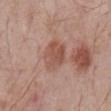Assessment: Recorded during total-body skin imaging; not selected for excision or biopsy. Acquisition and patient details: The lesion is on the abdomen. Automated image analysis of the tile measured a footprint of about 9.5 mm². And it measured a mean CIELAB color near L≈52 a*≈22 b*≈27, about 8 CIELAB-L* units darker than the surrounding skin, and a normalized border contrast of about 6.5. It also reported a classifier nevus-likeness of about 5/100 and a detector confidence of about 100 out of 100 that the crop contains a lesion. A roughly 15 mm field-of-view crop from a total-body skin photograph. The recorded lesion diameter is about 4 mm. A male subject about 70 years old. Captured under white-light illumination.